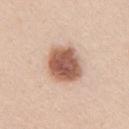biopsy status: no biopsy performed (imaged during a skin exam) | subject: female, aged approximately 20 | body site: the right upper arm | lighting: white-light | image source: ~15 mm crop, total-body skin-cancer survey.